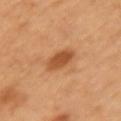An algorithmic analysis of the crop reported a footprint of about 6 mm², an eccentricity of roughly 0.7, and two-axis asymmetry of about 0.1. The software also gave a lesion color around L≈52 a*≈26 b*≈40 in CIELAB and roughly 12 lightness units darker than nearby skin. And it measured a border-irregularity index near 1/10, a within-lesion color-variation index near 2.5/10, and peripheral color asymmetry of about 1. The software also gave a nevus-likeness score of about 95/100 and a lesion-detection confidence of about 100/100. Cropped from a whole-body photographic skin survey; the tile spans about 15 mm. Captured under cross-polarized illumination. A female subject, aged 53–57. The lesion's longest dimension is about 3.5 mm. Located on the left upper arm.